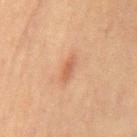Q: Is there a histopathology result?
A: catalogued during a skin exam; not biopsied
Q: How was this image acquired?
A: 15 mm crop, total-body photography
Q: What is the lesion's diameter?
A: ≈2.5 mm
Q: What lighting was used for the tile?
A: cross-polarized
Q: What is the anatomic site?
A: the abdomen
Q: What are the patient's age and sex?
A: male, aged 68–72
Q: Automated lesion metrics?
A: a lesion area of about 2.5 mm², a shape eccentricity near 0.9, and a shape-asymmetry score of about 0.3 (0 = symmetric); a border-irregularity index near 3/10 and a color-variation rating of about 0.5/10